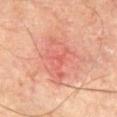The lesion was photographed on a routine skin check and not biopsied; there is no pathology result.
A male subject roughly 70 years of age.
Cropped from a whole-body photographic skin survey; the tile spans about 15 mm.
The tile uses cross-polarized illumination.
An algorithmic analysis of the crop reported a classifier nevus-likeness of about 0/100 and a lesion-detection confidence of about 100/100.
Located on the right thigh.
The lesion's longest dimension is about 6 mm.